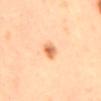{"lighting": "cross-polarized", "patient": {"sex": "female", "age_approx": 35}, "lesion_size": {"long_diameter_mm_approx": 2.5}, "image": {"source": "total-body photography crop", "field_of_view_mm": 15}, "site": "mid back"}